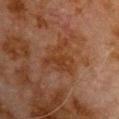The lesion was tiled from a total-body skin photograph and was not biopsied. On the front of the torso. A roughly 15 mm field-of-view crop from a total-body skin photograph. A male patient approximately 80 years of age.